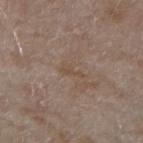Assessment: Captured during whole-body skin photography for melanoma surveillance; the lesion was not biopsied. Acquisition and patient details: Imaged with white-light lighting. Measured at roughly 3 mm in maximum diameter. From the arm. The patient is a female aged approximately 55. The lesion-visualizer software estimated a footprint of about 2.5 mm², an eccentricity of roughly 0.95, and two-axis asymmetry of about 0.45. And it measured a border-irregularity index near 5.5/10, a within-lesion color-variation index near 0/10, and peripheral color asymmetry of about 0. The analysis additionally found a nevus-likeness score of about 0/100 and lesion-presence confidence of about 100/100. A lesion tile, about 15 mm wide, cut from a 3D total-body photograph.